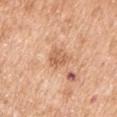This lesion was catalogued during total-body skin photography and was not selected for biopsy.
The lesion is located on the left upper arm.
A lesion tile, about 15 mm wide, cut from a 3D total-body photograph.
The patient is a male approximately 55 years of age.